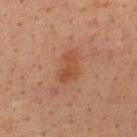No biopsy was performed on this lesion — it was imaged during a full skin examination and was not determined to be concerning. From the upper back. An algorithmic analysis of the crop reported an average lesion color of about L≈44 a*≈22 b*≈32 (CIELAB), a lesion–skin lightness drop of about 7, and a lesion-to-skin contrast of about 6.5 (normalized; higher = more distinct). A roughly 15 mm field-of-view crop from a total-body skin photograph. The subject is a male in their mid-30s. This is a cross-polarized tile. Measured at roughly 3.5 mm in maximum diameter.